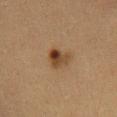Clinical impression:
Recorded during total-body skin imaging; not selected for excision or biopsy.
Context:
This is a cross-polarized tile. On the chest. This image is a 15 mm lesion crop taken from a total-body photograph. The subject is a female aged around 40.